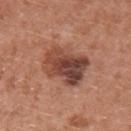Q: Was a biopsy performed?
A: imaged on a skin check; not biopsied
Q: How was this image acquired?
A: total-body-photography crop, ~15 mm field of view
Q: What did automated image analysis measure?
A: border irregularity of about 2 on a 0–10 scale, a within-lesion color-variation index near 9/10, and peripheral color asymmetry of about 3; a classifier nevus-likeness of about 45/100
Q: Patient demographics?
A: female, aged 38 to 42
Q: What is the lesion's diameter?
A: ~5.5 mm (longest diameter)
Q: Where on the body is the lesion?
A: the left upper arm